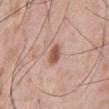Clinical impression:
The lesion was photographed on a routine skin check and not biopsied; there is no pathology result.
Background:
Cropped from a whole-body photographic skin survey; the tile spans about 15 mm. On the back. Automated tile analysis of the lesion measured a shape eccentricity near 0.7 and a symmetry-axis asymmetry near 0.15. It also reported roughly 12 lightness units darker than nearby skin and a lesion-to-skin contrast of about 8.5 (normalized; higher = more distinct). The analysis additionally found a border-irregularity index near 1.5/10 and peripheral color asymmetry of about 1. The analysis additionally found an automated nevus-likeness rating near 95 out of 100 and lesion-presence confidence of about 100/100. Longest diameter approximately 2.5 mm. The patient is a male in their mid- to late 50s.